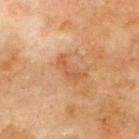Clinical impression:
Part of a total-body skin-imaging series; this lesion was reviewed on a skin check and was not flagged for biopsy.